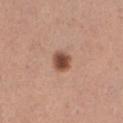Clinical impression:
Part of a total-body skin-imaging series; this lesion was reviewed on a skin check and was not flagged for biopsy.
Image and clinical context:
Captured under white-light illumination. On the leg. A 15 mm crop from a total-body photograph taken for skin-cancer surveillance. A female patient approximately 30 years of age.